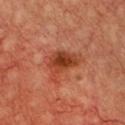{"biopsy_status": "not biopsied; imaged during a skin examination", "lesion_size": {"long_diameter_mm_approx": 4.5}, "site": "chest", "lighting": "cross-polarized", "automated_metrics": {"area_mm2_approx": 11.0, "shape_asymmetry": 0.45}, "patient": {"sex": "female", "age_approx": 45}, "image": {"source": "total-body photography crop", "field_of_view_mm": 15}}